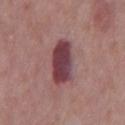- lighting: white-light
- subject: male, in their mid- to late 60s
- image source: total-body-photography crop, ~15 mm field of view
- lesion diameter: ~5 mm (longest diameter)
- body site: the chest